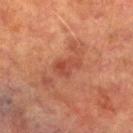follow-up: no biopsy performed (imaged during a skin exam) | patient: male, in their mid- to late 70s | acquisition: 15 mm crop, total-body photography | location: the left lower leg.